This image is a 15 mm lesion crop taken from a total-body photograph · the lesion is on the front of the torso · captured under cross-polarized illumination · a male subject approximately 60 years of age: 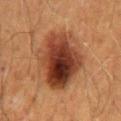Diagnosis:
Histopathologically confirmed as a melanoma in situ arising in association with a nevus (malignant).